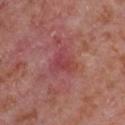Q: Was this lesion biopsied?
A: imaged on a skin check; not biopsied
Q: What is the imaging modality?
A: 15 mm crop, total-body photography
Q: How large is the lesion?
A: ~3.5 mm (longest diameter)
Q: How was the tile lit?
A: white-light illumination
Q: What is the anatomic site?
A: the chest
Q: What did automated image analysis measure?
A: an area of roughly 7 mm², an outline eccentricity of about 0.15 (0 = round, 1 = elongated), and a shape-asymmetry score of about 0.55 (0 = symmetric); a lesion color around L≈45 a*≈31 b*≈22 in CIELAB, about 7 CIELAB-L* units darker than the surrounding skin, and a lesion-to-skin contrast of about 5 (normalized; higher = more distinct); a classifier nevus-likeness of about 0/100 and lesion-presence confidence of about 95/100
Q: Who is the patient?
A: male, aged approximately 65